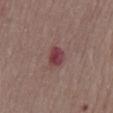workup: total-body-photography surveillance lesion; no biopsy
size: ~2.5 mm (longest diameter)
patient: male, in their mid-70s
body site: the abdomen
automated metrics: a shape-asymmetry score of about 0.2 (0 = symmetric); a lesion color around L≈40 a*≈26 b*≈17 in CIELAB, about 11 CIELAB-L* units darker than the surrounding skin, and a normalized border contrast of about 9; a border-irregularity rating of about 1.5/10, a within-lesion color-variation index near 4/10, and a peripheral color-asymmetry measure near 1
image: 15 mm crop, total-body photography
tile lighting: white-light